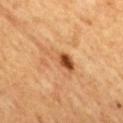The lesion was tiled from a total-body skin photograph and was not biopsied.
A 15 mm close-up tile from a total-body photography series done for melanoma screening.
A male subject, about 60 years old.
The lesion is located on the mid back.
An algorithmic analysis of the crop reported a mean CIELAB color near L≈54 a*≈24 b*≈39. And it measured a border-irregularity rating of about 5/10, a color-variation rating of about 10/10, and peripheral color asymmetry of about 6. The software also gave a classifier nevus-likeness of about 40/100 and a detector confidence of about 100 out of 100 that the crop contains a lesion.